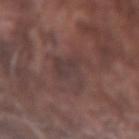Findings:
• workup · catalogued during a skin exam; not biopsied
• image · total-body-photography crop, ~15 mm field of view
• patient · male, aged approximately 75
• lighting · white-light illumination
• anatomic site · the left forearm
• diameter · ≈5 mm
• automated lesion analysis · an eccentricity of roughly 0.7